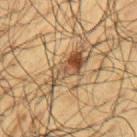Clinical impression: No biopsy was performed on this lesion — it was imaged during a full skin examination and was not determined to be concerning. Context: Cropped from a whole-body photographic skin survey; the tile spans about 15 mm. About 6.5 mm across. An algorithmic analysis of the crop reported an area of roughly 11 mm², an eccentricity of roughly 0.95, and two-axis asymmetry of about 0.45. It also reported border irregularity of about 7 on a 0–10 scale, a color-variation rating of about 9.5/10, and peripheral color asymmetry of about 3. A male subject about 60 years old. This is a cross-polarized tile. Located on the left upper arm.